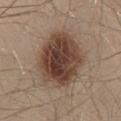{
  "biopsy_status": "not biopsied; imaged during a skin examination",
  "patient": {
    "sex": "male",
    "age_approx": 30
  },
  "site": "back",
  "lighting": "white-light",
  "image": {
    "source": "total-body photography crop",
    "field_of_view_mm": 15
  }
}